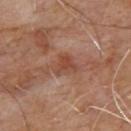Background: This is a cross-polarized tile. Longest diameter approximately 3 mm. The lesion-visualizer software estimated a footprint of about 5.5 mm², a shape eccentricity near 0.5, and two-axis asymmetry of about 0.4. It also reported a classifier nevus-likeness of about 0/100 and a lesion-detection confidence of about 100/100. A region of skin cropped from a whole-body photographic capture, roughly 15 mm wide. A male patient, approximately 65 years of age. On the upper back.